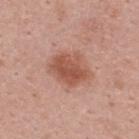| field | value |
|---|---|
| follow-up | total-body-photography surveillance lesion; no biopsy |
| patient | male, about 45 years old |
| image | 15 mm crop, total-body photography |
| lighting | white-light |
| diameter | ~5 mm (longest diameter) |
| site | the upper back |